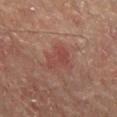Q: Was this lesion biopsied?
A: catalogued during a skin exam; not biopsied
Q: Lesion location?
A: the mid back
Q: What are the patient's age and sex?
A: male, about 65 years old
Q: How was this image acquired?
A: ~15 mm crop, total-body skin-cancer survey